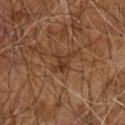Findings:
- biopsy status — no biopsy performed (imaged during a skin exam)
- acquisition — 15 mm crop, total-body photography
- size — about 2.5 mm
- TBP lesion metrics — an average lesion color of about L≈34 a*≈20 b*≈29 (CIELAB), about 7 CIELAB-L* units darker than the surrounding skin, and a normalized lesion–skin contrast near 7; border irregularity of about 4.5 on a 0–10 scale, a color-variation rating of about 3/10, and a peripheral color-asymmetry measure near 1; a classifier nevus-likeness of about 10/100
- illumination — cross-polarized illumination
- subject — male, about 60 years old
- site — the right arm